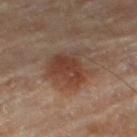The lesion was photographed on a routine skin check and not biopsied; there is no pathology result. A close-up tile cropped from a whole-body skin photograph, about 15 mm across. Imaged with cross-polarized lighting. A male subject in their mid- to late 80s or older. The lesion is on the right thigh.